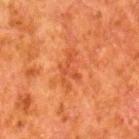Q: Is there a histopathology result?
A: total-body-photography surveillance lesion; no biopsy
Q: What lighting was used for the tile?
A: cross-polarized illumination
Q: Lesion size?
A: ≈4 mm
Q: Patient demographics?
A: male, aged 78 to 82
Q: Lesion location?
A: the leg
Q: Automated lesion metrics?
A: a lesion color around L≈42 a*≈29 b*≈36 in CIELAB and a lesion–skin lightness drop of about 6; internal color variation of about 0 on a 0–10 scale and peripheral color asymmetry of about 0
Q: What kind of image is this?
A: total-body-photography crop, ~15 mm field of view